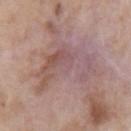Imaged during a routine full-body skin examination; the lesion was not biopsied and no histopathology is available. Located on the left upper arm. A roughly 15 mm field-of-view crop from a total-body skin photograph. A male patient, aged approximately 60.